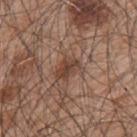{"biopsy_status": "not biopsied; imaged during a skin examination", "lighting": "white-light", "patient": {"sex": "male", "age_approx": 50}, "site": "upper back", "image": {"source": "total-body photography crop", "field_of_view_mm": 15}, "lesion_size": {"long_diameter_mm_approx": 3.5}}